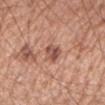workup — imaged on a skin check; not biopsied | image-analysis metrics — an average lesion color of about L≈52 a*≈23 b*≈28 (CIELAB), roughly 12 lightness units darker than nearby skin, and a normalized lesion–skin contrast near 8; border irregularity of about 2.5 on a 0–10 scale, a within-lesion color-variation index near 6/10, and peripheral color asymmetry of about 2.5; a classifier nevus-likeness of about 40/100 and a lesion-detection confidence of about 100/100 | anatomic site — the right upper arm | lesion size — ≈3 mm | image source — total-body-photography crop, ~15 mm field of view | illumination — white-light | patient — male, aged approximately 50.